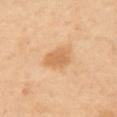Notes:
– biopsy status · no biopsy performed (imaged during a skin exam)
– subject · female, roughly 40 years of age
– image-analysis metrics · a mean CIELAB color near L≈68 a*≈20 b*≈41, a lesion–skin lightness drop of about 9, and a normalized lesion–skin contrast near 6; a nevus-likeness score of about 15/100 and a lesion-detection confidence of about 100/100
– lesion diameter · about 4 mm
– body site · the left upper arm
– image · ~15 mm tile from a whole-body skin photo